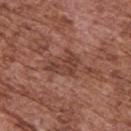Clinical impression: Captured during whole-body skin photography for melanoma surveillance; the lesion was not biopsied. Image and clinical context: From the upper back. This image is a 15 mm lesion crop taken from a total-body photograph. An algorithmic analysis of the crop reported a border-irregularity rating of about 6.5/10 and radial color variation of about 1. The analysis additionally found a classifier nevus-likeness of about 0/100. The tile uses white-light illumination. A male subject in their mid- to late 70s.